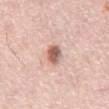The lesion was photographed on a routine skin check and not biopsied; there is no pathology result. A male patient, roughly 75 years of age. From the abdomen. This image is a 15 mm lesion crop taken from a total-body photograph.